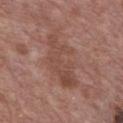biopsy_status: not biopsied; imaged during a skin examination